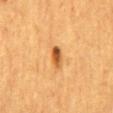<record>
<biopsy_status>not biopsied; imaged during a skin examination</biopsy_status>
<site>mid back</site>
<automated_metrics>
  <area_mm2_approx>4.0</area_mm2_approx>
  <eccentricity>0.85</eccentricity>
  <vs_skin_darker_L>13.0</vs_skin_darker_L>
  <vs_skin_contrast_norm>9.5</vs_skin_contrast_norm>
  <border_irregularity_0_10>3.0</border_irregularity_0_10>
  <color_variation_0_10>4.5</color_variation_0_10>
  <lesion_detection_confidence_0_100>100</lesion_detection_confidence_0_100>
</automated_metrics>
<image>
  <source>total-body photography crop</source>
  <field_of_view_mm>15</field_of_view_mm>
</image>
<lighting>cross-polarized</lighting>
<patient>
  <sex>female</sex>
  <age_approx>55</age_approx>
</patient>
</record>